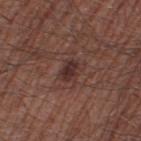Assessment:
Captured during whole-body skin photography for melanoma surveillance; the lesion was not biopsied.
Background:
Cropped from a total-body skin-imaging series; the visible field is about 15 mm. The patient is a male aged 63–67. From the right thigh.